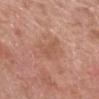biopsy_status: not biopsied; imaged during a skin examination
lighting: white-light
site: right lower leg
image:
  source: total-body photography crop
  field_of_view_mm: 15
automated_metrics:
  vs_skin_darker_L: 6.0
  vs_skin_contrast_norm: 4.5
  nevus_likeness_0_100: 0
  lesion_detection_confidence_0_100: 100
patient:
  sex: male
  age_approx: 80
lesion_size:
  long_diameter_mm_approx: 4.0